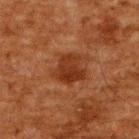Q: How was the tile lit?
A: cross-polarized illumination
Q: What kind of image is this?
A: ~15 mm tile from a whole-body skin photo
Q: Automated lesion metrics?
A: a lesion color around L≈26 a*≈23 b*≈29 in CIELAB, roughly 8 lightness units darker than nearby skin, and a lesion-to-skin contrast of about 8.5 (normalized; higher = more distinct); a border-irregularity rating of about 3/10, a color-variation rating of about 3.5/10, and radial color variation of about 1.5; an automated nevus-likeness rating near 50 out of 100 and lesion-presence confidence of about 100/100
Q: Lesion location?
A: the upper back
Q: Patient demographics?
A: male, aged approximately 60
Q: How large is the lesion?
A: ≈3.5 mm